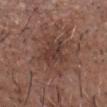Assessment: Part of a total-body skin-imaging series; this lesion was reviewed on a skin check and was not flagged for biopsy. Context: The lesion is on the head or neck. A male patient, aged 53–57. The tile uses white-light illumination. Longest diameter approximately 3.5 mm. Cropped from a total-body skin-imaging series; the visible field is about 15 mm.